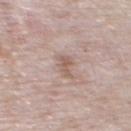biopsy_status: not biopsied; imaged during a skin examination
site: front of the torso
lesion_size:
  long_diameter_mm_approx: 3.5
lighting: white-light
image:
  source: total-body photography crop
  field_of_view_mm: 15
automated_metrics:
  area_mm2_approx: 4.5
  eccentricity: 0.9
patient:
  sex: male
  age_approx: 70